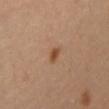imaging modality = 15 mm crop, total-body photography
lesion size = ~2 mm (longest diameter)
tile lighting = cross-polarized
anatomic site = the mid back
patient = female, aged 53 to 57